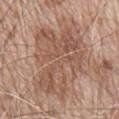  biopsy_status: not biopsied; imaged during a skin examination
  lighting: white-light
  image:
    source: total-body photography crop
    field_of_view_mm: 15
  patient:
    sex: male
    age_approx: 80
  site: mid back
  lesion_size:
    long_diameter_mm_approx: 10.0